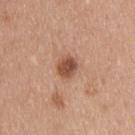Impression:
Recorded during total-body skin imaging; not selected for excision or biopsy.
Context:
The lesion's longest dimension is about 2.5 mm. Automated tile analysis of the lesion measured a lesion area of about 5 mm², a shape eccentricity near 0.55, and a symmetry-axis asymmetry near 0.15. Imaged with white-light lighting. A 15 mm crop from a total-body photograph taken for skin-cancer surveillance. A male patient, in their mid- to late 30s. The lesion is located on the upper back.